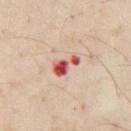– biopsy status: total-body-photography surveillance lesion; no biopsy
– patient: male, about 55 years old
– tile lighting: cross-polarized
– image source: total-body-photography crop, ~15 mm field of view
– site: the front of the torso
– lesion size: about 3 mm
– automated lesion analysis: a lesion color around L≈55 a*≈35 b*≈29 in CIELAB, roughly 18 lightness units darker than nearby skin, and a lesion-to-skin contrast of about 11.5 (normalized; higher = more distinct)